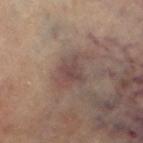workup=total-body-photography surveillance lesion; no biopsy | subject=female, roughly 65 years of age | image source=15 mm crop, total-body photography | site=the left thigh.